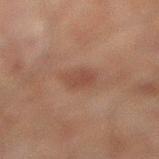Impression:
The lesion was tiled from a total-body skin photograph and was not biopsied.
Image and clinical context:
The lesion is on the left leg. A male patient, aged approximately 60. A 15 mm close-up tile from a total-body photography series done for melanoma screening.